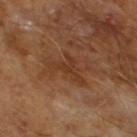This lesion was catalogued during total-body skin photography and was not selected for biopsy. A male subject aged 63 to 67. This image is a 15 mm lesion crop taken from a total-body photograph.